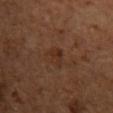Imaged during a routine full-body skin examination; the lesion was not biopsied and no histopathology is available. The lesion is on the upper back. A roughly 15 mm field-of-view crop from a total-body skin photograph. A female patient aged 58–62. The tile uses cross-polarized illumination. The total-body-photography lesion software estimated an area of roughly 3.5 mm², an outline eccentricity of about 0.8 (0 = round, 1 = elongated), and a symmetry-axis asymmetry near 0.35. It also reported a mean CIELAB color near L≈27 a*≈18 b*≈25 and a lesion-to-skin contrast of about 5.5 (normalized; higher = more distinct). The software also gave an automated nevus-likeness rating near 15 out of 100. The lesion's longest dimension is about 2.5 mm.